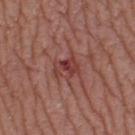Case summary:
– follow-up — no biopsy performed (imaged during a skin exam)
– lesion diameter — ~3 mm (longest diameter)
– lighting — white-light illumination
– image source — ~15 mm crop, total-body skin-cancer survey
– body site — the left lower leg
– automated metrics — a mean CIELAB color near L≈39 a*≈27 b*≈24, a lesion–skin lightness drop of about 9, and a normalized border contrast of about 7.5; a color-variation rating of about 3/10 and radial color variation of about 1
– subject — female, aged 38–42